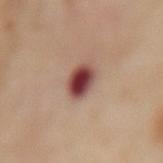Imaged during a routine full-body skin examination; the lesion was not biopsied and no histopathology is available. Located on the mid back. A female subject aged approximately 55. The recorded lesion diameter is about 3 mm. A roughly 15 mm field-of-view crop from a total-body skin photograph. The tile uses cross-polarized illumination.